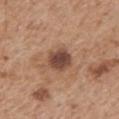Q: Was a biopsy performed?
A: imaged on a skin check; not biopsied
Q: Automated lesion metrics?
A: a lesion–skin lightness drop of about 13 and a normalized lesion–skin contrast near 10; a nevus-likeness score of about 35/100
Q: Illumination type?
A: white-light illumination
Q: What is the anatomic site?
A: the mid back
Q: What kind of image is this?
A: ~15 mm tile from a whole-body skin photo
Q: Lesion size?
A: about 3.5 mm
Q: Patient demographics?
A: male, aged 68–72